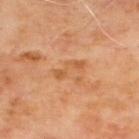Part of a total-body skin-imaging series; this lesion was reviewed on a skin check and was not flagged for biopsy. The lesion is located on the upper back. A lesion tile, about 15 mm wide, cut from a 3D total-body photograph. A male patient, approximately 65 years of age. Measured at roughly 3.5 mm in maximum diameter.